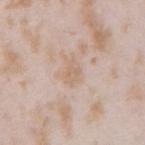diameter: ≈3 mm | tile lighting: white-light illumination | subject: female, in their mid-20s | TBP lesion metrics: an area of roughly 4.5 mm², an outline eccentricity of about 0.75 (0 = round, 1 = elongated), and two-axis asymmetry of about 0.35; a normalized lesion–skin contrast near 5; border irregularity of about 3 on a 0–10 scale, a within-lesion color-variation index near 1.5/10, and radial color variation of about 0.5; an automated nevus-likeness rating near 0 out of 100 and a detector confidence of about 100 out of 100 that the crop contains a lesion | site: the arm | image source: total-body-photography crop, ~15 mm field of view.